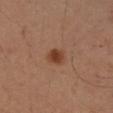{"biopsy_status": "not biopsied; imaged during a skin examination", "site": "right upper arm", "patient": {"sex": "female", "age_approx": 40}, "lesion_size": {"long_diameter_mm_approx": 2.5}, "lighting": "cross-polarized", "image": {"source": "total-body photography crop", "field_of_view_mm": 15}}